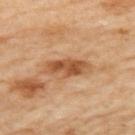notes — total-body-photography surveillance lesion; no biopsy
acquisition — ~15 mm crop, total-body skin-cancer survey
diameter — about 5.5 mm
tile lighting — cross-polarized illumination
subject — female, aged approximately 60
TBP lesion metrics — a footprint of about 9.5 mm², a shape eccentricity near 0.9, and a shape-asymmetry score of about 0.2 (0 = symmetric); an average lesion color of about L≈53 a*≈23 b*≈37 (CIELAB) and a normalized border contrast of about 8.5; a nevus-likeness score of about 5/100
body site — the left upper arm